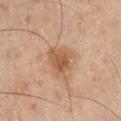Clinical impression: Captured during whole-body skin photography for melanoma surveillance; the lesion was not biopsied. Background: A 15 mm close-up extracted from a 3D total-body photography capture. The patient is a male about 45 years old. This is a cross-polarized tile. The lesion is on the right lower leg.